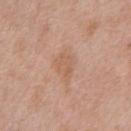Assessment:
Recorded during total-body skin imaging; not selected for excision or biopsy.
Acquisition and patient details:
Imaged with white-light lighting. The total-body-photography lesion software estimated a lesion area of about 6 mm² and two-axis asymmetry of about 0.35. The analysis additionally found a lesion-detection confidence of about 100/100. From the abdomen. Measured at roughly 3.5 mm in maximum diameter. A 15 mm close-up tile from a total-body photography series done for melanoma screening. A male subject aged 48 to 52.